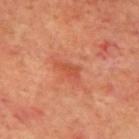Impression:
Imaged during a routine full-body skin examination; the lesion was not biopsied and no histopathology is available.
Acquisition and patient details:
Located on the mid back. Captured under cross-polarized illumination. A 15 mm close-up tile from a total-body photography series done for melanoma screening. The patient is a male about 70 years old. Automated tile analysis of the lesion measured an area of roughly 3 mm² and a shape-asymmetry score of about 0.35 (0 = symmetric). It also reported a lesion–skin lightness drop of about 7 and a lesion-to-skin contrast of about 5.5 (normalized; higher = more distinct). The analysis additionally found a border-irregularity index near 3.5/10, a color-variation rating of about 1/10, and peripheral color asymmetry of about 0.5. It also reported a detector confidence of about 100 out of 100 that the crop contains a lesion. Longest diameter approximately 3 mm.